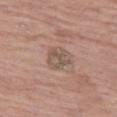Q: Where on the body is the lesion?
A: the left thigh
Q: Who is the patient?
A: female, aged around 85 or older
Q: What is the imaging modality?
A: total-body-photography crop, ~15 mm field of view